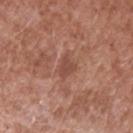Recorded during total-body skin imaging; not selected for excision or biopsy.
An algorithmic analysis of the crop reported an outline eccentricity of about 0.75 (0 = round, 1 = elongated) and a symmetry-axis asymmetry near 0.4. It also reported a lesion color around L≈48 a*≈23 b*≈27 in CIELAB, a lesion–skin lightness drop of about 8, and a normalized border contrast of about 6.5. And it measured a border-irregularity index near 3.5/10, a color-variation rating of about 0.5/10, and peripheral color asymmetry of about 0. It also reported a classifier nevus-likeness of about 0/100 and a lesion-detection confidence of about 100/100.
A male subject aged around 55.
This image is a 15 mm lesion crop taken from a total-body photograph.
The lesion is on the left upper arm.
Imaged with white-light lighting.
Longest diameter approximately 3 mm.